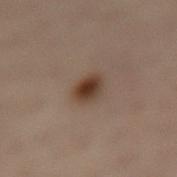Findings:
- body site: the lower back
- TBP lesion metrics: a mean CIELAB color near L≈31 a*≈14 b*≈22 and roughly 10 lightness units darker than nearby skin; border irregularity of about 2 on a 0–10 scale, a within-lesion color-variation index near 4.5/10, and a peripheral color-asymmetry measure near 1.5
- image: ~15 mm crop, total-body skin-cancer survey
- subject: female, aged 53–57
- illumination: cross-polarized
- size: ≈3.5 mm